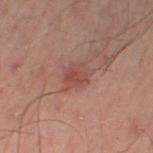Part of a total-body skin-imaging series; this lesion was reviewed on a skin check and was not flagged for biopsy. A close-up tile cropped from a whole-body skin photograph, about 15 mm across. The tile uses cross-polarized illumination. Automated tile analysis of the lesion measured a border-irregularity rating of about 2/10 and a within-lesion color-variation index near 2.5/10. It also reported a classifier nevus-likeness of about 25/100 and a lesion-detection confidence of about 100/100. The patient is a male aged around 50. Longest diameter approximately 3 mm. The lesion is on the left leg.